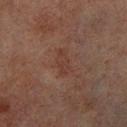{
  "image": {
    "source": "total-body photography crop",
    "field_of_view_mm": 15
  },
  "site": "right lower leg",
  "patient": {
    "sex": "male",
    "age_approx": 70
  }
}